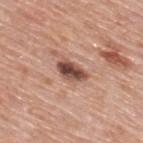Assessment: Recorded during total-body skin imaging; not selected for excision or biopsy. Background: The tile uses white-light illumination. The patient is a male roughly 80 years of age. Automated tile analysis of the lesion measured an average lesion color of about L≈48 a*≈22 b*≈26 (CIELAB), a lesion–skin lightness drop of about 17, and a normalized lesion–skin contrast near 11.5. And it measured a classifier nevus-likeness of about 45/100 and a lesion-detection confidence of about 100/100. On the upper back. A 15 mm close-up tile from a total-body photography series done for melanoma screening. About 3.5 mm across.